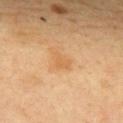<case>
<biopsy_status>not biopsied; imaged during a skin examination</biopsy_status>
<patient>
  <sex>female</sex>
  <age_approx>45</age_approx>
</patient>
<site>upper back</site>
<lighting>cross-polarized</lighting>
<lesion_size>
  <long_diameter_mm_approx>2.5</long_diameter_mm_approx>
</lesion_size>
<image>
  <source>total-body photography crop</source>
  <field_of_view_mm>15</field_of_view_mm>
</image>
</case>